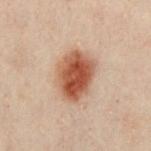{"biopsy_status": "not biopsied; imaged during a skin examination", "lesion_size": {"long_diameter_mm_approx": 5.5}, "patient": {"sex": "male", "age_approx": 50}, "image": {"source": "total-body photography crop", "field_of_view_mm": 15}, "site": "chest"}